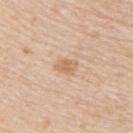| field | value |
|---|---|
| workup | catalogued during a skin exam; not biopsied |
| site | the upper back |
| lighting | white-light |
| patient | female, about 50 years old |
| automated lesion analysis | an average lesion color of about L≈65 a*≈19 b*≈34 (CIELAB), a lesion–skin lightness drop of about 9, and a normalized border contrast of about 6.5; a nevus-likeness score of about 5/100 and lesion-presence confidence of about 100/100 |
| acquisition | ~15 mm tile from a whole-body skin photo |
| lesion diameter | about 2.5 mm |